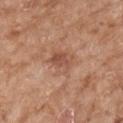<case>
<biopsy_status>not biopsied; imaged during a skin examination</biopsy_status>
<image>
  <source>total-body photography crop</source>
  <field_of_view_mm>15</field_of_view_mm>
</image>
<lighting>white-light</lighting>
<patient>
  <sex>female</sex>
  <age_approx>75</age_approx>
</patient>
<site>arm</site>
<lesion_size>
  <long_diameter_mm_approx>4.0</long_diameter_mm_approx>
</lesion_size>
</case>